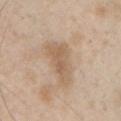Impression: Imaged during a routine full-body skin examination; the lesion was not biopsied and no histopathology is available. Context: The lesion is on the left upper arm. Approximately 5.5 mm at its widest. A close-up tile cropped from a whole-body skin photograph, about 15 mm across. Automated image analysis of the tile measured a mean CIELAB color near L≈59 a*≈16 b*≈31. And it measured a classifier nevus-likeness of about 0/100 and a detector confidence of about 100 out of 100 that the crop contains a lesion. A male patient aged 48 to 52. Imaged with white-light lighting.